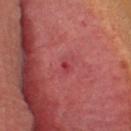Q: Is there a histopathology result?
A: no biopsy performed (imaged during a skin exam)